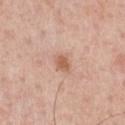biopsy_status: not biopsied; imaged during a skin examination
lighting: white-light
site: chest
patient:
  sex: male
  age_approx: 60
lesion_size:
  long_diameter_mm_approx: 2.5
image:
  source: total-body photography crop
  field_of_view_mm: 15
automated_metrics:
  vs_skin_contrast_norm: 7.0
  color_variation_0_10: 2.0
  peripheral_color_asymmetry: 0.5
  nevus_likeness_0_100: 50
  lesion_detection_confidence_0_100: 100